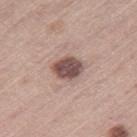Assessment:
No biopsy was performed on this lesion — it was imaged during a full skin examination and was not determined to be concerning.
Background:
About 3.5 mm across. Automated tile analysis of the lesion measured a lesion area of about 8 mm², an outline eccentricity of about 0.6 (0 = round, 1 = elongated), and two-axis asymmetry of about 0.15. The analysis additionally found a mean CIELAB color near L≈50 a*≈18 b*≈21 and a normalized lesion–skin contrast near 11. The patient is a male aged approximately 65. Located on the right thigh. A lesion tile, about 15 mm wide, cut from a 3D total-body photograph. The tile uses white-light illumination.